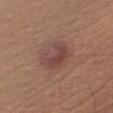<case>
  <biopsy_status>not biopsied; imaged during a skin examination</biopsy_status>
  <image>
    <source>total-body photography crop</source>
    <field_of_view_mm>15</field_of_view_mm>
  </image>
  <patient>
    <sex>female</sex>
    <age_approx>65</age_approx>
  </patient>
  <site>right thigh</site>
</case>